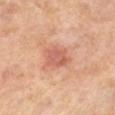The lesion was photographed on a routine skin check and not biopsied; there is no pathology result. The lesion-visualizer software estimated a border-irregularity rating of about 3.5/10, internal color variation of about 3.5 on a 0–10 scale, and a peripheral color-asymmetry measure near 1.5. The software also gave a classifier nevus-likeness of about 25/100 and lesion-presence confidence of about 100/100. Measured at roughly 4 mm in maximum diameter. The lesion is on the leg. A female subject, in their mid- to late 50s. A region of skin cropped from a whole-body photographic capture, roughly 15 mm wide. The tile uses cross-polarized illumination.